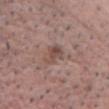| key | value |
|---|---|
| notes | imaged on a skin check; not biopsied |
| anatomic site | the chest |
| illumination | white-light illumination |
| patient | male, approximately 70 years of age |
| image source | total-body-photography crop, ~15 mm field of view |
| lesion diameter | ~3 mm (longest diameter) |